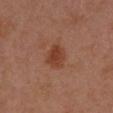biopsy status: total-body-photography surveillance lesion; no biopsy | diameter: ~3 mm (longest diameter) | site: the left forearm | imaging modality: total-body-photography crop, ~15 mm field of view | subject: female, aged 38–42 | automated metrics: an outline eccentricity of about 0.25 (0 = round, 1 = elongated); a classifier nevus-likeness of about 95/100 and a detector confidence of about 100 out of 100 that the crop contains a lesion | lighting: cross-polarized illumination.